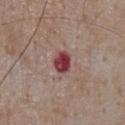{"biopsy_status": "not biopsied; imaged during a skin examination", "site": "front of the torso", "patient": {"sex": "male", "age_approx": 75}, "image": {"source": "total-body photography crop", "field_of_view_mm": 15}}